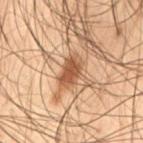Assessment: This lesion was catalogued during total-body skin photography and was not selected for biopsy. Clinical summary: A male patient, aged 48–52. A 15 mm close-up tile from a total-body photography series done for melanoma screening. The lesion is on the left thigh.